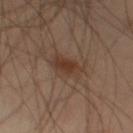biopsy status — imaged on a skin check; not biopsied
automated metrics — a lesion area of about 7.5 mm², an eccentricity of roughly 0.65, and a shape-asymmetry score of about 0.25 (0 = symmetric); a mean CIELAB color near L≈37 a*≈17 b*≈26, a lesion–skin lightness drop of about 8, and a normalized border contrast of about 7; a border-irregularity index near 3/10, a within-lesion color-variation index near 3.5/10, and peripheral color asymmetry of about 1; a classifier nevus-likeness of about 90/100 and a detector confidence of about 100 out of 100 that the crop contains a lesion
acquisition — ~15 mm crop, total-body skin-cancer survey
lighting — cross-polarized illumination
subject — male, approximately 40 years of age
size — about 3.5 mm
anatomic site — the left thigh